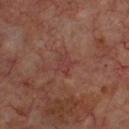Findings:
* follow-up: catalogued during a skin exam; not biopsied
* image source: 15 mm crop, total-body photography
* subject: male, approximately 70 years of age
* automated lesion analysis: a mean CIELAB color near L≈36 a*≈23 b*≈22, roughly 5 lightness units darker than nearby skin, and a lesion-to-skin contrast of about 5 (normalized; higher = more distinct); a border-irregularity index near 3/10, a within-lesion color-variation index near 1/10, and a peripheral color-asymmetry measure near 0.5; a lesion-detection confidence of about 100/100
* size: ≈2.5 mm
* body site: the chest